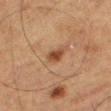Recorded during total-body skin imaging; not selected for excision or biopsy.
A male patient aged around 75.
A 15 mm close-up tile from a total-body photography series done for melanoma screening.
The lesion is on the leg.